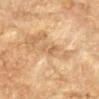biopsy status: catalogued during a skin exam; not biopsied | imaging modality: ~15 mm tile from a whole-body skin photo | TBP lesion metrics: a lesion area of about 4 mm², a shape eccentricity near 0.85, and two-axis asymmetry of about 0.25; a classifier nevus-likeness of about 0/100 and a lesion-detection confidence of about 55/100 | patient: male, aged 83 or over | tile lighting: cross-polarized | body site: the left forearm.